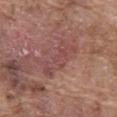Recorded during total-body skin imaging; not selected for excision or biopsy. The subject is a male aged 73 to 77. From the front of the torso. About 5.5 mm across. A 15 mm close-up tile from a total-body photography series done for melanoma screening.